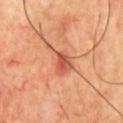This lesion was catalogued during total-body skin photography and was not selected for biopsy. A lesion tile, about 15 mm wide, cut from a 3D total-body photograph. The subject is a male aged 68–72. Imaged with cross-polarized lighting. The lesion is on the chest. Approximately 4.5 mm at its widest.